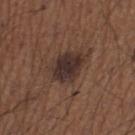This lesion was catalogued during total-body skin photography and was not selected for biopsy.
The recorded lesion diameter is about 4 mm.
A male subject in their mid- to late 60s.
Captured under white-light illumination.
The lesion is on the left thigh.
A roughly 15 mm field-of-view crop from a total-body skin photograph.
Automated tile analysis of the lesion measured a footprint of about 11 mm² and a symmetry-axis asymmetry near 0.15. The software also gave a classifier nevus-likeness of about 45/100 and a detector confidence of about 100 out of 100 that the crop contains a lesion.